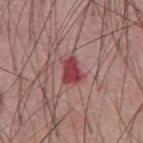Clinical summary: On the abdomen. A close-up tile cropped from a whole-body skin photograph, about 15 mm across. About 3 mm across. The patient is a male about 55 years old.